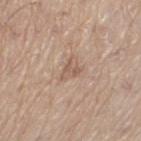Assessment:
Captured during whole-body skin photography for melanoma surveillance; the lesion was not biopsied.
Image and clinical context:
About 3 mm across. The patient is a male aged 68 to 72. The tile uses white-light illumination. The lesion is located on the left thigh. A 15 mm close-up tile from a total-body photography series done for melanoma screening.